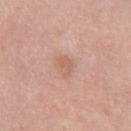Imaged during a routine full-body skin examination; the lesion was not biopsied and no histopathology is available. A female patient aged around 50. The lesion is on the right thigh. A close-up tile cropped from a whole-body skin photograph, about 15 mm across. Approximately 2.5 mm at its widest.